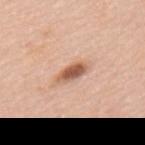Notes:
* workup · imaged on a skin check; not biopsied
* body site · the back
* imaging modality · ~15 mm crop, total-body skin-cancer survey
* automated lesion analysis · an average lesion color of about L≈59 a*≈23 b*≈32 (CIELAB) and a lesion-to-skin contrast of about 9.5 (normalized; higher = more distinct); a border-irregularity rating of about 3/10, internal color variation of about 4.5 on a 0–10 scale, and peripheral color asymmetry of about 1; a nevus-likeness score of about 95/100 and lesion-presence confidence of about 100/100
* subject · male, aged approximately 80
* lesion size · ≈4 mm
* illumination · white-light